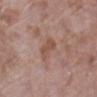Findings:
* biopsy status: imaged on a skin check; not biopsied